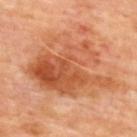Q: Was this lesion biopsied?
A: imaged on a skin check; not biopsied
Q: What kind of image is this?
A: total-body-photography crop, ~15 mm field of view
Q: Who is the patient?
A: male, roughly 65 years of age
Q: Lesion location?
A: the upper back